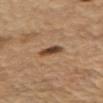notes: no biopsy performed (imaged during a skin exam)
TBP lesion metrics: a footprint of about 4 mm² and an eccentricity of roughly 0.8; an average lesion color of about L≈45 a*≈19 b*≈32 (CIELAB); a border-irregularity index near 2.5/10, internal color variation of about 5 on a 0–10 scale, and peripheral color asymmetry of about 1.5
tile lighting: white-light illumination
site: the arm
lesion diameter: about 2.5 mm
patient: male, aged approximately 70
acquisition: 15 mm crop, total-body photography